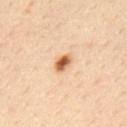Clinical impression: Imaged during a routine full-body skin examination; the lesion was not biopsied and no histopathology is available. Acquisition and patient details: The tile uses cross-polarized illumination. On the upper back. This image is a 15 mm lesion crop taken from a total-body photograph. The patient is a male aged approximately 35.